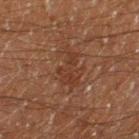Assessment: The lesion was tiled from a total-body skin photograph and was not biopsied. Acquisition and patient details: A lesion tile, about 15 mm wide, cut from a 3D total-body photograph. Approximately 5.5 mm at its widest. The total-body-photography lesion software estimated a border-irregularity rating of about 5.5/10. Imaged with cross-polarized lighting. A male subject, aged 58 to 62. From the left thigh.